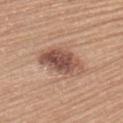Impression:
Part of a total-body skin-imaging series; this lesion was reviewed on a skin check and was not flagged for biopsy.
Context:
The lesion is on the left upper arm. Longest diameter approximately 6 mm. This image is a 15 mm lesion crop taken from a total-body photograph. A female patient, aged 63–67. Automated image analysis of the tile measured a lesion color around L≈52 a*≈21 b*≈27 in CIELAB, roughly 13 lightness units darker than nearby skin, and a normalized lesion–skin contrast near 9. It also reported a classifier nevus-likeness of about 80/100. Captured under white-light illumination.